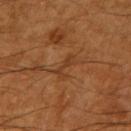Clinical impression: This lesion was catalogued during total-body skin photography and was not selected for biopsy. Background: The lesion is located on the right upper arm. This is a cross-polarized tile. A male subject, about 60 years old. A 15 mm close-up extracted from a 3D total-body photography capture.